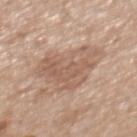Notes:
• workup · imaged on a skin check; not biopsied
• illumination · white-light illumination
• image · 15 mm crop, total-body photography
• anatomic site · the mid back
• size · about 6.5 mm
• patient · male, roughly 70 years of age
• TBP lesion metrics · a lesion–skin lightness drop of about 8 and a lesion-to-skin contrast of about 5.5 (normalized; higher = more distinct); border irregularity of about 5 on a 0–10 scale and a color-variation rating of about 4/10